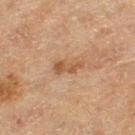biopsy status: catalogued during a skin exam; not biopsied | subject: female, in their mid-50s | diameter: ~3.5 mm (longest diameter) | location: the left thigh | image: ~15 mm crop, total-body skin-cancer survey | tile lighting: cross-polarized.